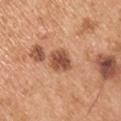This lesion was catalogued during total-body skin photography and was not selected for biopsy. A 15 mm close-up tile from a total-body photography series done for melanoma screening. A male patient, in their mid-50s. The lesion's longest dimension is about 3.5 mm. The lesion is located on the right upper arm. The total-body-photography lesion software estimated a lesion area of about 7 mm² and a shape eccentricity near 0.7. The analysis additionally found a border-irregularity index near 1.5/10, internal color variation of about 3.5 on a 0–10 scale, and radial color variation of about 1. The analysis additionally found an automated nevus-likeness rating near 25 out of 100 and a lesion-detection confidence of about 100/100. Captured under white-light illumination.